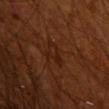The lesion was tiled from a total-body skin photograph and was not biopsied.
This is a cross-polarized tile.
A male subject approximately 70 years of age.
Cropped from a whole-body photographic skin survey; the tile spans about 15 mm.
On the arm.
The lesion's longest dimension is about 3 mm.
The lesion-visualizer software estimated a footprint of about 5 mm², an outline eccentricity of about 0.6 (0 = round, 1 = elongated), and two-axis asymmetry of about 0.55. And it measured an automated nevus-likeness rating near 0 out of 100 and a lesion-detection confidence of about 100/100.